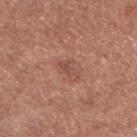| feature | finding |
|---|---|
| biopsy status | no biopsy performed (imaged during a skin exam) |
| image source | total-body-photography crop, ~15 mm field of view |
| tile lighting | white-light |
| body site | the left lower leg |
| patient | male, aged 68 to 72 |